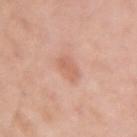Q: Where on the body is the lesion?
A: the right upper arm
Q: What is the imaging modality?
A: total-body-photography crop, ~15 mm field of view
Q: Who is the patient?
A: female, aged approximately 40
Q: How large is the lesion?
A: ≈2.5 mm
Q: What did automated image analysis measure?
A: border irregularity of about 2.5 on a 0–10 scale, a within-lesion color-variation index near 1.5/10, and radial color variation of about 0.5; a nevus-likeness score of about 20/100 and a lesion-detection confidence of about 100/100
Q: What lighting was used for the tile?
A: white-light illumination